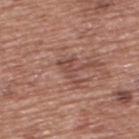The lesion was photographed on a routine skin check and not biopsied; there is no pathology result. This is a white-light tile. The lesion's longest dimension is about 4 mm. A male subject roughly 75 years of age. A lesion tile, about 15 mm wide, cut from a 3D total-body photograph. Located on the upper back.